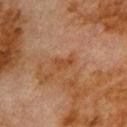The lesion was photographed on a routine skin check and not biopsied; there is no pathology result. The patient is a male roughly 80 years of age. An algorithmic analysis of the crop reported a lesion color around L≈35 a*≈20 b*≈30 in CIELAB, about 5 CIELAB-L* units darker than the surrounding skin, and a normalized lesion–skin contrast near 6.5. The software also gave a within-lesion color-variation index near 1/10 and peripheral color asymmetry of about 0.5. It also reported a classifier nevus-likeness of about 0/100. Captured under cross-polarized illumination. On the upper back. Cropped from a whole-body photographic skin survey; the tile spans about 15 mm. About 2.5 mm across.